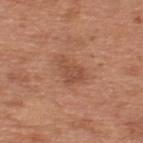<record>
<biopsy_status>not biopsied; imaged during a skin examination</biopsy_status>
<patient>
  <sex>male</sex>
  <age_approx>65</age_approx>
</patient>
<image>
  <source>total-body photography crop</source>
  <field_of_view_mm>15</field_of_view_mm>
</image>
<site>upper back</site>
<lesion_size>
  <long_diameter_mm_approx>4.0</long_diameter_mm_approx>
</lesion_size>
<lighting>white-light</lighting>
<automated_metrics>
  <cielab_L>50</cielab_L>
  <cielab_a>23</cielab_a>
  <cielab_b>32</cielab_b>
  <vs_skin_contrast_norm>5.5</vs_skin_contrast_norm>
  <border_irregularity_0_10>4.5</border_irregularity_0_10>
  <color_variation_0_10>2.5</color_variation_0_10>
</automated_metrics>
</record>